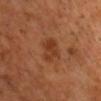Q: What is the imaging modality?
A: total-body-photography crop, ~15 mm field of view
Q: How large is the lesion?
A: about 3.5 mm
Q: Who is the patient?
A: male, about 50 years old
Q: What is the anatomic site?
A: the head or neck
Q: How was the tile lit?
A: cross-polarized illumination
Q: What did automated image analysis measure?
A: an area of roughly 6.5 mm², a shape eccentricity near 0.75, and two-axis asymmetry of about 0.3; about 8 CIELAB-L* units darker than the surrounding skin and a normalized lesion–skin contrast near 6.5; a border-irregularity index near 3/10, a within-lesion color-variation index near 4/10, and peripheral color asymmetry of about 1.5